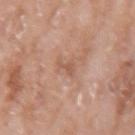| key | value |
|---|---|
| biopsy status | imaged on a skin check; not biopsied |
| body site | the right forearm |
| subject | female, approximately 75 years of age |
| image source | ~15 mm crop, total-body skin-cancer survey |